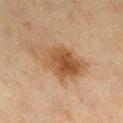The lesion was photographed on a routine skin check and not biopsied; there is no pathology result. The lesion-visualizer software estimated an area of roughly 18 mm² and an eccentricity of roughly 0.8. And it measured a border-irregularity rating of about 5/10, internal color variation of about 5 on a 0–10 scale, and peripheral color asymmetry of about 2. It also reported a detector confidence of about 100 out of 100 that the crop contains a lesion. Cropped from a whole-body photographic skin survey; the tile spans about 15 mm. Longest diameter approximately 6.5 mm. Imaged with cross-polarized lighting. From the right lower leg. The subject is a female in their 40s.